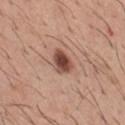This lesion was catalogued during total-body skin photography and was not selected for biopsy.
The total-body-photography lesion software estimated a lesion area of about 6 mm², a shape eccentricity near 0.65, and a shape-asymmetry score of about 0.25 (0 = symmetric). The analysis additionally found a lesion color around L≈47 a*≈22 b*≈26 in CIELAB, about 16 CIELAB-L* units darker than the surrounding skin, and a normalized lesion–skin contrast near 11.
About 3 mm across.
Located on the back.
A roughly 15 mm field-of-view crop from a total-body skin photograph.
A male subject, about 40 years old.
This is a white-light tile.